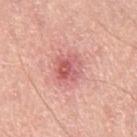A male subject aged approximately 50.
A 15 mm close-up tile from a total-body photography series done for melanoma screening.
The recorded lesion diameter is about 3.5 mm.
The lesion is located on the right thigh.
Captured under white-light illumination.